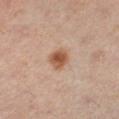workup: imaged on a skin check; not biopsied
lesion size: ~2.5 mm (longest diameter)
patient: female, aged approximately 40
automated lesion analysis: a mean CIELAB color near L≈55 a*≈22 b*≈32, about 13 CIELAB-L* units darker than the surrounding skin, and a normalized lesion–skin contrast near 9; a border-irregularity index near 2.5/10, a within-lesion color-variation index near 3.5/10, and a peripheral color-asymmetry measure near 1; an automated nevus-likeness rating near 100 out of 100 and a lesion-detection confidence of about 100/100
image: ~15 mm crop, total-body skin-cancer survey
body site: the leg